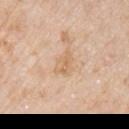workup: total-body-photography surveillance lesion; no biopsy
illumination: white-light
site: the arm
image source: total-body-photography crop, ~15 mm field of view
size: about 3 mm
patient: male, aged around 75